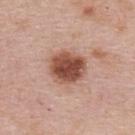biopsy status = total-body-photography surveillance lesion; no biopsy
patient = female, aged 48 to 52
imaging modality = 15 mm crop, total-body photography
site = the upper back